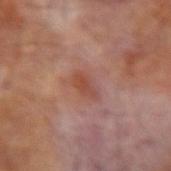biopsy status=catalogued during a skin exam; not biopsied | patient=male, approximately 65 years of age | site=the right forearm | acquisition=15 mm crop, total-body photography.